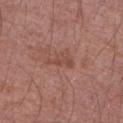notes = total-body-photography surveillance lesion; no biopsy
image source = total-body-photography crop, ~15 mm field of view
site = the left upper arm
patient = male, aged around 50
lighting = white-light illumination